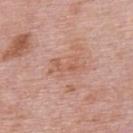Captured during whole-body skin photography for melanoma surveillance; the lesion was not biopsied.
The tile uses white-light illumination.
The patient is a male aged approximately 75.
The lesion is located on the upper back.
A 15 mm close-up tile from a total-body photography series done for melanoma screening.